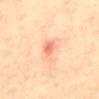Part of a total-body skin-imaging series; this lesion was reviewed on a skin check and was not flagged for biopsy. A female patient aged around 45. On the abdomen. Longest diameter approximately 3.5 mm. Captured under cross-polarized illumination. A 15 mm close-up extracted from a 3D total-body photography capture.